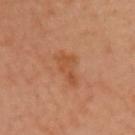Imaged during a routine full-body skin examination; the lesion was not biopsied and no histopathology is available.
The patient is a female aged around 60.
The lesion-visualizer software estimated a lesion color around L≈44 a*≈22 b*≈33 in CIELAB and a normalized lesion–skin contrast near 6. It also reported a color-variation rating of about 1/10 and peripheral color asymmetry of about 0.5.
On the chest.
The lesion's longest dimension is about 4 mm.
Cropped from a total-body skin-imaging series; the visible field is about 15 mm.
Imaged with cross-polarized lighting.